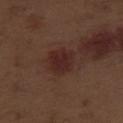No biopsy was performed on this lesion — it was imaged during a full skin examination and was not determined to be concerning.
From the right thigh.
A roughly 15 mm field-of-view crop from a total-body skin photograph.
The patient is a male aged 68–72.
Automated image analysis of the tile measured a mean CIELAB color near L≈26 a*≈20 b*≈21, a lesion–skin lightness drop of about 7, and a lesion-to-skin contrast of about 8 (normalized; higher = more distinct).
The recorded lesion diameter is about 3.5 mm.
Captured under white-light illumination.